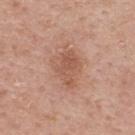Impression: Part of a total-body skin-imaging series; this lesion was reviewed on a skin check and was not flagged for biopsy.